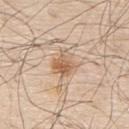Clinical summary: The lesion is on the upper back. A male patient aged around 80. Longest diameter approximately 3 mm. A 15 mm crop from a total-body photograph taken for skin-cancer surveillance. Captured under white-light illumination.